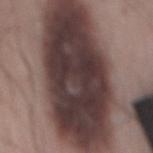Findings:
• biopsy status — imaged on a skin check; not biopsied
• anatomic site — the mid back
• TBP lesion metrics — a footprint of about 90 mm² and a shape-asymmetry score of about 0.1 (0 = symmetric); a border-irregularity rating of about 2/10; a classifier nevus-likeness of about 0/100 and lesion-presence confidence of about 5/100
• lighting — white-light illumination
• subject — male, aged approximately 50
• image source — 15 mm crop, total-body photography